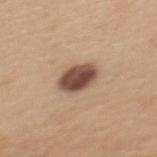• notes · no biopsy performed (imaged during a skin exam)
• imaging modality · 15 mm crop, total-body photography
• illumination · white-light
• body site · the upper back
• lesion size · ≈4 mm
• patient · female, approximately 30 years of age